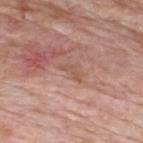Imaged during a routine full-body skin examination; the lesion was not biopsied and no histopathology is available.
A region of skin cropped from a whole-body photographic capture, roughly 15 mm wide.
The tile uses white-light illumination.
From the upper back.
A male subject, aged 58–62.
The total-body-photography lesion software estimated a mean CIELAB color near L≈54 a*≈21 b*≈28, a lesion–skin lightness drop of about 7, and a normalized border contrast of about 5.5. The software also gave a border-irregularity index near 5.5/10, a within-lesion color-variation index near 0/10, and radial color variation of about 0.
Approximately 2.5 mm at its widest.